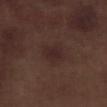Findings:
* biopsy status: catalogued during a skin exam; not biopsied
* site: the right lower leg
* automated metrics: an outline eccentricity of about 0.6 (0 = round, 1 = elongated) and two-axis asymmetry of about 0.25; a mean CIELAB color near L≈25 a*≈17 b*≈17, roughly 5 lightness units darker than nearby skin, and a normalized border contrast of about 7; a classifier nevus-likeness of about 40/100 and a lesion-detection confidence of about 100/100
* patient: male, aged around 70
* image: total-body-photography crop, ~15 mm field of view
* illumination: white-light
* diameter: ~3 mm (longest diameter)